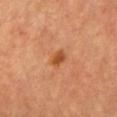Q: Was this lesion biopsied?
A: total-body-photography surveillance lesion; no biopsy
Q: What are the patient's age and sex?
A: female, aged approximately 50
Q: What is the anatomic site?
A: the chest
Q: Automated lesion metrics?
A: a border-irregularity rating of about 2/10, internal color variation of about 2 on a 0–10 scale, and a peripheral color-asymmetry measure near 1
Q: What kind of image is this?
A: 15 mm crop, total-body photography
Q: Illumination type?
A: cross-polarized